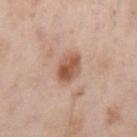Assessment:
The lesion was photographed on a routine skin check and not biopsied; there is no pathology result.
Context:
A 15 mm close-up extracted from a 3D total-body photography capture. A male subject aged 53–57. Located on the left upper arm. Captured under white-light illumination. The total-body-photography lesion software estimated a color-variation rating of about 5.5/10 and a peripheral color-asymmetry measure near 2. The analysis additionally found a classifier nevus-likeness of about 80/100 and lesion-presence confidence of about 100/100.